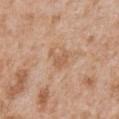Assessment: The lesion was photographed on a routine skin check and not biopsied; there is no pathology result. Acquisition and patient details: Imaged with white-light lighting. The lesion is located on the chest. A male patient roughly 65 years of age. A lesion tile, about 15 mm wide, cut from a 3D total-body photograph. Approximately 3 mm at its widest.